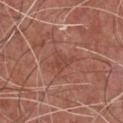follow-up: no biopsy performed (imaged during a skin exam)
location: the chest
size: about 3 mm
acquisition: 15 mm crop, total-body photography
patient: male, in their mid- to late 70s
illumination: white-light
automated metrics: an area of roughly 4.5 mm², a shape eccentricity near 0.65, and two-axis asymmetry of about 0.45; a nevus-likeness score of about 0/100